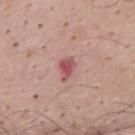| feature | finding |
|---|---|
| notes | imaged on a skin check; not biopsied |
| subject | male, aged 33 to 37 |
| lesion size | ≈2.5 mm |
| site | the mid back |
| acquisition | ~15 mm tile from a whole-body skin photo |
| lighting | white-light illumination |
| image-analysis metrics | a lesion area of about 3.5 mm², an eccentricity of roughly 0.75, and a shape-asymmetry score of about 0.35 (0 = symmetric); a border-irregularity rating of about 3/10, a within-lesion color-variation index near 2.5/10, and peripheral color asymmetry of about 0.5 |